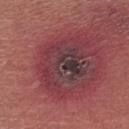Background: Measured at roughly 6 mm in maximum diameter. The lesion-visualizer software estimated an average lesion color of about L≈36 a*≈22 b*≈14 (CIELAB), roughly 7 lightness units darker than nearby skin, and a normalized border contrast of about 8. The software also gave an automated nevus-likeness rating near 0 out of 100 and lesion-presence confidence of about 95/100. This image is a 15 mm lesion crop taken from a total-body photograph. A male subject aged 63–67. The lesion is located on the leg.